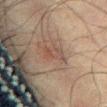  biopsy_status: not biopsied; imaged during a skin examination
  lighting: cross-polarized
  patient:
    sex: male
    age_approx: 70
  lesion_size:
    long_diameter_mm_approx: 3.5
  image:
    source: total-body photography crop
    field_of_view_mm: 15
  site: abdomen